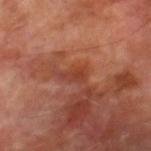Impression: The lesion was tiled from a total-body skin photograph and was not biopsied. Clinical summary: Imaged with cross-polarized lighting. Located on the right lower leg. The lesion-visualizer software estimated a lesion area of about 3.5 mm², a shape eccentricity near 0.85, and a symmetry-axis asymmetry near 0.4. The analysis additionally found a lesion color around L≈40 a*≈28 b*≈31 in CIELAB and a normalized border contrast of about 5.5. The analysis additionally found a border-irregularity index near 4/10, a color-variation rating of about 2.5/10, and radial color variation of about 1. The software also gave a nevus-likeness score of about 0/100 and a detector confidence of about 100 out of 100 that the crop contains a lesion. A male patient aged approximately 70. Measured at roughly 3 mm in maximum diameter. A 15 mm close-up tile from a total-body photography series done for melanoma screening.